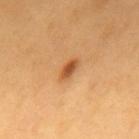On the upper back.
A male subject, about 60 years old.
Measured at roughly 3 mm in maximum diameter.
A lesion tile, about 15 mm wide, cut from a 3D total-body photograph.
The tile uses cross-polarized illumination.
Automated tile analysis of the lesion measured a lesion area of about 3.5 mm², an outline eccentricity of about 0.9 (0 = round, 1 = elongated), and two-axis asymmetry of about 0.3. The analysis additionally found a classifier nevus-likeness of about 95/100.